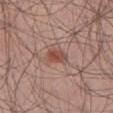No biopsy was performed on this lesion — it was imaged during a full skin examination and was not determined to be concerning.